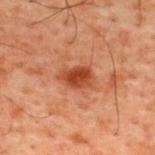The lesion was photographed on a routine skin check and not biopsied; there is no pathology result.